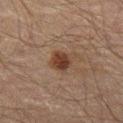Impression: No biopsy was performed on this lesion — it was imaged during a full skin examination and was not determined to be concerning. Background: Automated tile analysis of the lesion measured an area of roughly 5 mm², a shape eccentricity near 0.5, and two-axis asymmetry of about 0.15. About 2.5 mm across. The tile uses cross-polarized illumination. On the left thigh. A close-up tile cropped from a whole-body skin photograph, about 15 mm across. A male patient, roughly 60 years of age.